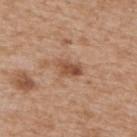Recorded during total-body skin imaging; not selected for excision or biopsy.
The lesion is located on the upper back.
A male patient aged 63–67.
A close-up tile cropped from a whole-body skin photograph, about 15 mm across.
Measured at roughly 3 mm in maximum diameter.
Captured under white-light illumination.